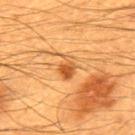biopsy_status: not biopsied; imaged during a skin examination
image:
  source: total-body photography crop
  field_of_view_mm: 15
patient:
  sex: male
  age_approx: 60
site: mid back